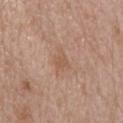This lesion was catalogued during total-body skin photography and was not selected for biopsy. Located on the upper back. A region of skin cropped from a whole-body photographic capture, roughly 15 mm wide. The recorded lesion diameter is about 3 mm. Imaged with white-light lighting. The patient is a female in their mid-40s.